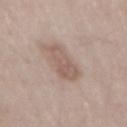Captured during whole-body skin photography for melanoma surveillance; the lesion was not biopsied.
The subject is a male aged 53–57.
Longest diameter approximately 5.5 mm.
This image is a 15 mm lesion crop taken from a total-body photograph.
From the mid back.